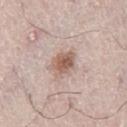<record>
  <patient>
    <sex>male</sex>
    <age_approx>65</age_approx>
  </patient>
  <lighting>white-light</lighting>
  <automated_metrics>
    <area_mm2_approx>7.0</area_mm2_approx>
    <shape_asymmetry>0.2</shape_asymmetry>
    <border_irregularity_0_10>2.5</border_irregularity_0_10>
    <color_variation_0_10>4.5</color_variation_0_10>
    <peripheral_color_asymmetry>1.5</peripheral_color_asymmetry>
    <nevus_likeness_0_100>80</nevus_likeness_0_100>
    <lesion_detection_confidence_0_100>100</lesion_detection_confidence_0_100>
  </automated_metrics>
  <site>right thigh</site>
  <image>
    <source>total-body photography crop</source>
    <field_of_view_mm>15</field_of_view_mm>
  </image>
  <lesion_size>
    <long_diameter_mm_approx>3.5</long_diameter_mm_approx>
  </lesion_size>
</record>